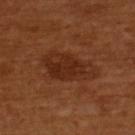Findings:
- follow-up: total-body-photography surveillance lesion; no biopsy
- body site: the upper back
- patient: female, aged around 55
- image source: 15 mm crop, total-body photography
- automated lesion analysis: a lesion–skin lightness drop of about 7
- size: ≈6.5 mm